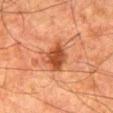| feature | finding |
|---|---|
| workup | total-body-photography surveillance lesion; no biopsy |
| lighting | cross-polarized |
| anatomic site | the right thigh |
| acquisition | ~15 mm crop, total-body skin-cancer survey |
| TBP lesion metrics | a footprint of about 9.5 mm², a shape eccentricity near 0.7, and a symmetry-axis asymmetry near 0.3; a mean CIELAB color near L≈40 a*≈25 b*≈32, a lesion–skin lightness drop of about 11, and a normalized border contrast of about 9 |
| patient | male, aged approximately 80 |
| size | ~4 mm (longest diameter) |